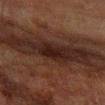Recorded during total-body skin imaging; not selected for excision or biopsy. The subject is a male aged approximately 65. A 15 mm crop from a total-body photograph taken for skin-cancer surveillance. The lesion is located on the left forearm. Measured at roughly 2.5 mm in maximum diameter. Imaged with cross-polarized lighting.